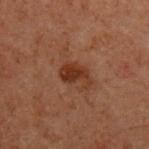Part of a total-body skin-imaging series; this lesion was reviewed on a skin check and was not flagged for biopsy.
A male subject aged approximately 60.
A close-up tile cropped from a whole-body skin photograph, about 15 mm across.
The lesion-visualizer software estimated a shape eccentricity near 0.8 and a symmetry-axis asymmetry near 0.3. It also reported a mean CIELAB color near L≈25 a*≈19 b*≈25, a lesion–skin lightness drop of about 8, and a normalized lesion–skin contrast near 8.5.
About 3.5 mm across.
Located on the chest.
This is a cross-polarized tile.